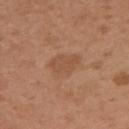| feature | finding |
|---|---|
| notes | total-body-photography surveillance lesion; no biopsy |
| patient | female, aged 28–32 |
| image source | 15 mm crop, total-body photography |
| body site | the left upper arm |